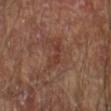workup — total-body-photography surveillance lesion; no biopsy | body site — the right forearm | image source — ~15 mm crop, total-body skin-cancer survey | patient — male, aged 63–67 | tile lighting — cross-polarized illumination.